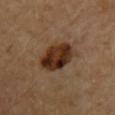Q: Was this lesion biopsied?
A: total-body-photography surveillance lesion; no biopsy